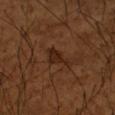Recorded during total-body skin imaging; not selected for excision or biopsy.
Measured at roughly 3.5 mm in maximum diameter.
Automated tile analysis of the lesion measured a mean CIELAB color near L≈24 a*≈19 b*≈27 and roughly 7 lightness units darker than nearby skin. It also reported a classifier nevus-likeness of about 0/100 and lesion-presence confidence of about 100/100.
A close-up tile cropped from a whole-body skin photograph, about 15 mm across.
Captured under cross-polarized illumination.
A male patient roughly 65 years of age.
The lesion is on the right upper arm.